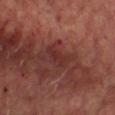Recorded during total-body skin imaging; not selected for excision or biopsy. A patient aged 63–67. A 15 mm close-up tile from a total-body photography series done for melanoma screening. This is a cross-polarized tile. About 4 mm across. The lesion is located on the front of the torso.